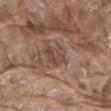No biopsy was performed on this lesion — it was imaged during a full skin examination and was not determined to be concerning. A male subject, aged around 80. This is a white-light tile. Automated tile analysis of the lesion measured a lesion area of about 7 mm² and an eccentricity of roughly 0.7. The software also gave internal color variation of about 4 on a 0–10 scale and radial color variation of about 1.5. The software also gave an automated nevus-likeness rating near 0 out of 100 and lesion-presence confidence of about 60/100. The lesion is on the abdomen. A lesion tile, about 15 mm wide, cut from a 3D total-body photograph.